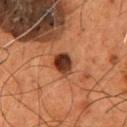Captured during whole-body skin photography for melanoma surveillance; the lesion was not biopsied. The lesion is on the chest. A region of skin cropped from a whole-body photographic capture, roughly 15 mm wide. A male subject, aged around 55.